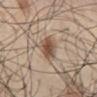Assessment:
Imaged during a routine full-body skin examination; the lesion was not biopsied and no histopathology is available.
Acquisition and patient details:
Longest diameter approximately 3.5 mm. The lesion is on the front of the torso. A male subject, about 30 years old. A region of skin cropped from a whole-body photographic capture, roughly 15 mm wide. Imaged with white-light lighting.